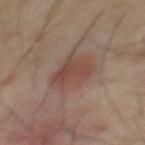Part of a total-body skin-imaging series; this lesion was reviewed on a skin check and was not flagged for biopsy. A male subject, about 70 years old. From the front of the torso. A lesion tile, about 15 mm wide, cut from a 3D total-body photograph.